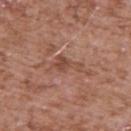Assessment: The lesion was photographed on a routine skin check and not biopsied; there is no pathology result. Background: Captured under white-light illumination. The lesion's longest dimension is about 4 mm. A close-up tile cropped from a whole-body skin photograph, about 15 mm across. The total-body-photography lesion software estimated a lesion–skin lightness drop of about 8 and a normalized lesion–skin contrast near 6. The analysis additionally found a border-irregularity index near 6/10 and internal color variation of about 2.5 on a 0–10 scale. Located on the back. The subject is a male in their mid- to late 50s.